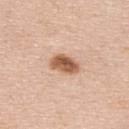Q: Was a biopsy performed?
A: total-body-photography surveillance lesion; no biopsy
Q: What are the patient's age and sex?
A: male, aged approximately 45
Q: What kind of image is this?
A: 15 mm crop, total-body photography
Q: Lesion location?
A: the upper back